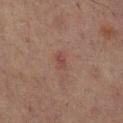notes: no biopsy performed (imaged during a skin exam) | size: ≈2.5 mm | body site: the mid back | image source: 15 mm crop, total-body photography | subject: male, approximately 65 years of age.